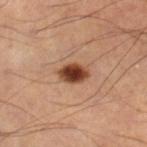<record>
<biopsy_status>not biopsied; imaged during a skin examination</biopsy_status>
<patient>
  <sex>male</sex>
  <age_approx>50</age_approx>
</patient>
<image>
  <source>total-body photography crop</source>
  <field_of_view_mm>15</field_of_view_mm>
</image>
<site>left leg</site>
<lighting>cross-polarized</lighting>
</record>